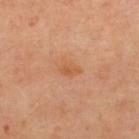body site = the upper back | subject = female, in their 40s | TBP lesion metrics = an area of roughly 4 mm² and a shape eccentricity near 0.75; an average lesion color of about L≈59 a*≈25 b*≈39 (CIELAB) and about 7 CIELAB-L* units darker than the surrounding skin; internal color variation of about 2 on a 0–10 scale and peripheral color asymmetry of about 0.5; a nevus-likeness score of about 5/100 | lighting = cross-polarized illumination | size = ~2.5 mm (longest diameter) | image = total-body-photography crop, ~15 mm field of view.